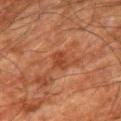| field | value |
|---|---|
| workup | total-body-photography surveillance lesion; no biopsy |
| illumination | cross-polarized |
| body site | the right thigh |
| acquisition | total-body-photography crop, ~15 mm field of view |
| patient | male, aged 78 to 82 |